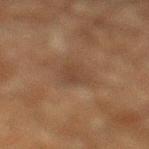Captured during whole-body skin photography for melanoma surveillance; the lesion was not biopsied.
Measured at roughly 3 mm in maximum diameter.
This is a cross-polarized tile.
A male subject roughly 60 years of age.
Automated tile analysis of the lesion measured about 5 CIELAB-L* units darker than the surrounding skin and a normalized lesion–skin contrast near 5. The analysis additionally found a border-irregularity index near 3/10, internal color variation of about 2 on a 0–10 scale, and radial color variation of about 0.5.
On the left lower leg.
A 15 mm close-up tile from a total-body photography series done for melanoma screening.